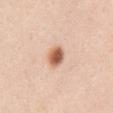Assessment: Captured during whole-body skin photography for melanoma surveillance; the lesion was not biopsied. Image and clinical context: Cropped from a total-body skin-imaging series; the visible field is about 15 mm. The lesion-visualizer software estimated a mean CIELAB color near L≈62 a*≈23 b*≈33, a lesion–skin lightness drop of about 16, and a lesion-to-skin contrast of about 10.5 (normalized; higher = more distinct). The patient is a female approximately 40 years of age. On the chest. Captured under white-light illumination.